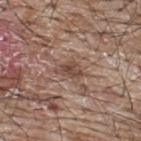Q: What is the lesion's diameter?
A: ~3 mm (longest diameter)
Q: What lighting was used for the tile?
A: white-light
Q: What kind of image is this?
A: ~15 mm crop, total-body skin-cancer survey
Q: Where on the body is the lesion?
A: the upper back
Q: What did automated image analysis measure?
A: a lesion area of about 4 mm² and a shape eccentricity near 0.8; a lesion color around L≈46 a*≈18 b*≈25 in CIELAB, about 10 CIELAB-L* units darker than the surrounding skin, and a normalized border contrast of about 7.5; a border-irregularity index near 4/10, a color-variation rating of about 3/10, and a peripheral color-asymmetry measure near 1
Q: Who is the patient?
A: male, in their mid- to late 60s